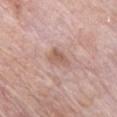biopsy status: total-body-photography surveillance lesion; no biopsy
tile lighting: white-light
patient: male, approximately 75 years of age
diameter: about 3 mm
image source: ~15 mm crop, total-body skin-cancer survey
TBP lesion metrics: a footprint of about 4 mm² and a symmetry-axis asymmetry near 0.4; an average lesion color of about L≈58 a*≈20 b*≈27 (CIELAB), roughly 9 lightness units darker than nearby skin, and a lesion-to-skin contrast of about 6.5 (normalized; higher = more distinct); a border-irregularity index near 3.5/10; a lesion-detection confidence of about 100/100
site: the chest